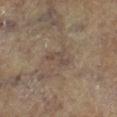The lesion was tiled from a total-body skin photograph and was not biopsied.
Approximately 3 mm at its widest.
The subject is a male aged around 85 or older.
Located on the right lower leg.
This image is a 15 mm lesion crop taken from a total-body photograph.
This is a cross-polarized tile.